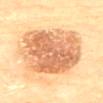Clinical impression: No biopsy was performed on this lesion — it was imaged during a full skin examination and was not determined to be concerning. Background: A female subject, aged around 80. Cropped from a total-body skin-imaging series; the visible field is about 15 mm. The tile uses cross-polarized illumination. On the mid back. An algorithmic analysis of the crop reported a lesion area of about 49 mm², an eccentricity of roughly 0.75, and two-axis asymmetry of about 0.15. The analysis additionally found a border-irregularity rating of about 2/10, a within-lesion color-variation index near 6/10, and radial color variation of about 2. It also reported a nevus-likeness score of about 65/100 and a lesion-detection confidence of about 100/100. About 10.5 mm across.